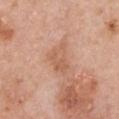Recorded during total-body skin imaging; not selected for excision or biopsy. The lesion is located on the chest. A 15 mm close-up extracted from a 3D total-body photography capture. The lesion's longest dimension is about 4 mm. The subject is a female about 60 years old.